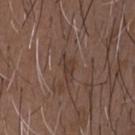The lesion was photographed on a routine skin check and not biopsied; there is no pathology result.
The recorded lesion diameter is about 3.5 mm.
Cropped from a total-body skin-imaging series; the visible field is about 15 mm.
The patient is a male aged 48–52.
Located on the chest.
Imaged with white-light lighting.